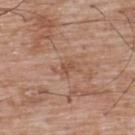Recorded during total-body skin imaging; not selected for excision or biopsy. The lesion is located on the upper back. Cropped from a total-body skin-imaging series; the visible field is about 15 mm. A male subject, about 50 years old. This is a white-light tile. An algorithmic analysis of the crop reported a border-irregularity index near 4.5/10 and a peripheral color-asymmetry measure near 0.